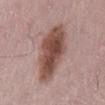follow-up — no biopsy performed (imaged during a skin exam); site — the lower back; patient — male, roughly 55 years of age; image source — 15 mm crop, total-body photography.